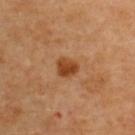Impression: No biopsy was performed on this lesion — it was imaged during a full skin examination and was not determined to be concerning. Background: From the back. A region of skin cropped from a whole-body photographic capture, roughly 15 mm wide. A male patient, aged 43–47.